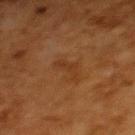Assessment: Recorded during total-body skin imaging; not selected for excision or biopsy. Image and clinical context: A female subject, about 50 years old. A 15 mm close-up tile from a total-body photography series done for melanoma screening. Located on the upper back.